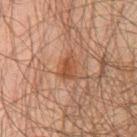Impression:
Recorded during total-body skin imaging; not selected for excision or biopsy.
Context:
On the chest. This image is a 15 mm lesion crop taken from a total-body photograph. The patient is a male about 60 years old. Imaged with cross-polarized lighting. Automated image analysis of the tile measured a shape eccentricity near 0.75 and a symmetry-axis asymmetry near 0.35. The analysis additionally found an average lesion color of about L≈37 a*≈19 b*≈27 (CIELAB), roughly 7 lightness units darker than nearby skin, and a normalized lesion–skin contrast near 7. It also reported radial color variation of about 0.5. It also reported an automated nevus-likeness rating near 25 out of 100.